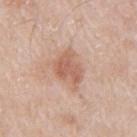size — ~4.5 mm (longest diameter)
subject — male, aged 63–67
body site — the arm
image source — ~15 mm crop, total-body skin-cancer survey
tile lighting — white-light
image-analysis metrics — an area of roughly 10 mm², an outline eccentricity of about 0.7 (0 = round, 1 = elongated), and a symmetry-axis asymmetry near 0.3; a mean CIELAB color near L≈60 a*≈21 b*≈29, a lesion–skin lightness drop of about 10, and a normalized border contrast of about 6.5; a classifier nevus-likeness of about 20/100 and a lesion-detection confidence of about 100/100